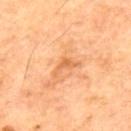Assessment:
The lesion was photographed on a routine skin check and not biopsied; there is no pathology result.
Clinical summary:
The lesion is located on the upper back. Automated image analysis of the tile measured an average lesion color of about L≈62 a*≈26 b*≈42 (CIELAB), about 9 CIELAB-L* units darker than the surrounding skin, and a normalized border contrast of about 6. The analysis additionally found border irregularity of about 6 on a 0–10 scale, a color-variation rating of about 1.5/10, and radial color variation of about 0.5. Imaged with cross-polarized lighting. The subject is a male aged 53–57. A 15 mm crop from a total-body photograph taken for skin-cancer surveillance. Longest diameter approximately 3 mm.